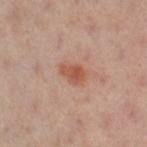Findings:
• notes — no biopsy performed (imaged during a skin exam)
• diameter — ~3 mm (longest diameter)
• subject — female, aged 38–42
• illumination — cross-polarized illumination
• body site — the left leg
• TBP lesion metrics — an average lesion color of about L≈54 a*≈24 b*≈30 (CIELAB) and roughly 9 lightness units darker than nearby skin; a nevus-likeness score of about 80/100 and lesion-presence confidence of about 100/100
• imaging modality — total-body-photography crop, ~15 mm field of view